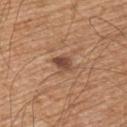Recorded during total-body skin imaging; not selected for excision or biopsy.
The tile uses white-light illumination.
A male subject, aged 63 to 67.
From the left upper arm.
An algorithmic analysis of the crop reported an area of roughly 3.5 mm² and a shape eccentricity near 0.7. It also reported a lesion-to-skin contrast of about 9 (normalized; higher = more distinct). The analysis additionally found a border-irregularity index near 2/10, internal color variation of about 3.5 on a 0–10 scale, and a peripheral color-asymmetry measure near 1.5. And it measured an automated nevus-likeness rating near 65 out of 100 and a lesion-detection confidence of about 100/100.
Measured at roughly 2.5 mm in maximum diameter.
Cropped from a total-body skin-imaging series; the visible field is about 15 mm.